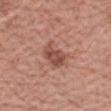Part of a total-body skin-imaging series; this lesion was reviewed on a skin check and was not flagged for biopsy.
Located on the left forearm.
Cropped from a total-body skin-imaging series; the visible field is about 15 mm.
The patient is a female in their 70s.